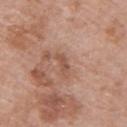Assessment: Imaged during a routine full-body skin examination; the lesion was not biopsied and no histopathology is available. Clinical summary: About 2.5 mm across. The patient is a male aged 68–72. Located on the upper back. A 15 mm close-up extracted from a 3D total-body photography capture. Captured under white-light illumination.